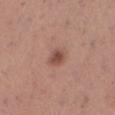biopsy status — catalogued during a skin exam; not biopsied | TBP lesion metrics — about 11 CIELAB-L* units darker than the surrounding skin and a normalized border contrast of about 7.5; internal color variation of about 3.5 on a 0–10 scale; a lesion-detection confidence of about 100/100 | tile lighting — white-light | patient — female, aged 28–32 | anatomic site — the left lower leg | diameter — about 2.5 mm | image — ~15 mm tile from a whole-body skin photo.